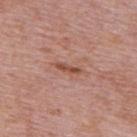Assessment:
This lesion was catalogued during total-body skin photography and was not selected for biopsy.
Context:
The lesion is on the upper back. A 15 mm crop from a total-body photograph taken for skin-cancer surveillance. A male subject in their mid-70s.